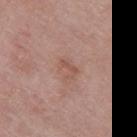Q: Is there a histopathology result?
A: catalogued during a skin exam; not biopsied
Q: Lesion size?
A: ≈3 mm
Q: What is the imaging modality?
A: 15 mm crop, total-body photography
Q: Illumination type?
A: white-light illumination
Q: What is the anatomic site?
A: the left thigh
Q: What are the patient's age and sex?
A: female, about 70 years old
Q: What did automated image analysis measure?
A: roughly 7 lightness units darker than nearby skin and a lesion-to-skin contrast of about 5 (normalized; higher = more distinct); internal color variation of about 0.5 on a 0–10 scale